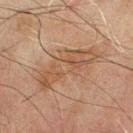| feature | finding |
|---|---|
| follow-up | total-body-photography surveillance lesion; no biopsy |
| lighting | cross-polarized illumination |
| diameter | ≈8.5 mm |
| body site | the chest |
| image source | ~15 mm tile from a whole-body skin photo |
| automated metrics | border irregularity of about 5 on a 0–10 scale, internal color variation of about 4.5 on a 0–10 scale, and a peripheral color-asymmetry measure near 1.5 |
| subject | male, about 70 years old |